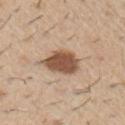Part of a total-body skin-imaging series; this lesion was reviewed on a skin check and was not flagged for biopsy. The lesion is located on the right upper arm. This image is a 15 mm lesion crop taken from a total-body photograph. The patient is a male approximately 60 years of age.